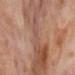Impression: Imaged during a routine full-body skin examination; the lesion was not biopsied and no histopathology is available. Clinical summary: A lesion tile, about 15 mm wide, cut from a 3D total-body photograph. A female subject aged 53–57. Imaged with cross-polarized lighting. About 10.5 mm across. The lesion is on the right lower leg.